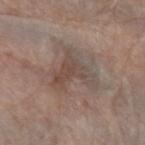Clinical impression: No biopsy was performed on this lesion — it was imaged during a full skin examination and was not determined to be concerning. Acquisition and patient details: Imaged with white-light lighting. A 15 mm close-up tile from a total-body photography series done for melanoma screening. From the arm. A female subject, about 65 years old. An algorithmic analysis of the crop reported border irregularity of about 8 on a 0–10 scale and internal color variation of about 5 on a 0–10 scale. And it measured a classifier nevus-likeness of about 0/100 and a lesion-detection confidence of about 85/100. The recorded lesion diameter is about 5.5 mm.